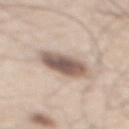  biopsy_status: not biopsied; imaged during a skin examination
  image:
    source: total-body photography crop
    field_of_view_mm: 15
  lesion_size:
    long_diameter_mm_approx: 5.5
  patient:
    sex: male
    age_approx: 60
  lighting: white-light
  site: mid back